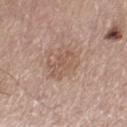No biopsy was performed on this lesion — it was imaged during a full skin examination and was not determined to be concerning.
Longest diameter approximately 3 mm.
Automated tile analysis of the lesion measured a lesion area of about 5 mm², a shape eccentricity near 0.75, and a shape-asymmetry score of about 0.3 (0 = symmetric).
A male patient, roughly 65 years of age.
On the left thigh.
Cropped from a total-body skin-imaging series; the visible field is about 15 mm.